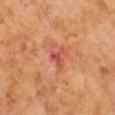Clinical impression: The lesion was photographed on a routine skin check and not biopsied; there is no pathology result. Context: The subject is a male roughly 60 years of age. Located on the right arm. This image is a 15 mm lesion crop taken from a total-body photograph.